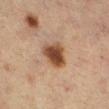Cropped from a whole-body photographic skin survey; the tile spans about 15 mm. The recorded lesion diameter is about 4 mm. A female patient, aged around 55. The tile uses cross-polarized illumination. The lesion is located on the right lower leg. The lesion-visualizer software estimated a lesion color around L≈37 a*≈17 b*≈26 in CIELAB, about 13 CIELAB-L* units darker than the surrounding skin, and a normalized border contrast of about 11. The software also gave an automated nevus-likeness rating near 100 out of 100.